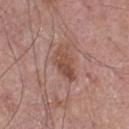– workup: total-body-photography surveillance lesion; no biopsy
– acquisition: 15 mm crop, total-body photography
– site: the chest
– subject: male, approximately 75 years of age
– lesion diameter: about 4 mm
– illumination: white-light